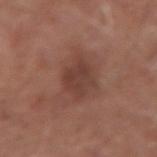Captured during whole-body skin photography for melanoma surveillance; the lesion was not biopsied.
Cropped from a whole-body photographic skin survey; the tile spans about 15 mm.
A male patient, roughly 65 years of age.
About 4 mm across.
From the left forearm.
Automated image analysis of the tile measured an average lesion color of about L≈40 a*≈21 b*≈24 (CIELAB), roughly 8 lightness units darker than nearby skin, and a lesion-to-skin contrast of about 6.5 (normalized; higher = more distinct). It also reported border irregularity of about 2.5 on a 0–10 scale, a within-lesion color-variation index near 2/10, and peripheral color asymmetry of about 0.5. The analysis additionally found an automated nevus-likeness rating near 5 out of 100 and a lesion-detection confidence of about 100/100.